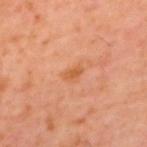Clinical impression: This lesion was catalogued during total-body skin photography and was not selected for biopsy. Context: A male subject approximately 60 years of age. The lesion-visualizer software estimated a classifier nevus-likeness of about 0/100 and a lesion-detection confidence of about 100/100. A roughly 15 mm field-of-view crop from a total-body skin photograph. The lesion is on the mid back.